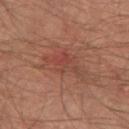| key | value |
|---|---|
| notes | total-body-photography surveillance lesion; no biopsy |
| illumination | cross-polarized illumination |
| automated lesion analysis | a footprint of about 10 mm² and a shape-asymmetry score of about 0.45 (0 = symmetric); an average lesion color of about L≈43 a*≈24 b*≈27 (CIELAB), roughly 6 lightness units darker than nearby skin, and a lesion-to-skin contrast of about 5 (normalized; higher = more distinct); border irregularity of about 6 on a 0–10 scale, a color-variation rating of about 5.5/10, and radial color variation of about 2; lesion-presence confidence of about 100/100 |
| subject | male, aged around 65 |
| image source | ~15 mm tile from a whole-body skin photo |
| anatomic site | the left thigh |
| size | ~5.5 mm (longest diameter) |